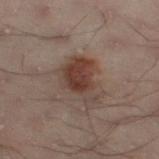A lesion tile, about 15 mm wide, cut from a 3D total-body photograph. The lesion is on the left lower leg. A male patient aged 48 to 52.